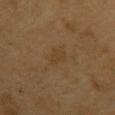The lesion was tiled from a total-body skin photograph and was not biopsied. Captured under cross-polarized illumination. A male subject roughly 60 years of age. The lesion is on the front of the torso. The lesion's longest dimension is about 3 mm. A 15 mm close-up tile from a total-body photography series done for melanoma screening.